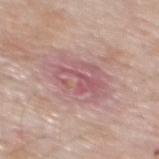Assessment:
This lesion was catalogued during total-body skin photography and was not selected for biopsy.
Clinical summary:
Cropped from a total-body skin-imaging series; the visible field is about 15 mm. A male subject, about 60 years old. Automated tile analysis of the lesion measured a footprint of about 19 mm², a shape eccentricity near 0.7, and a shape-asymmetry score of about 0.2 (0 = symmetric). It also reported a lesion–skin lightness drop of about 8 and a lesion-to-skin contrast of about 6 (normalized; higher = more distinct). The analysis additionally found a border-irregularity index near 2.5/10 and a within-lesion color-variation index near 7/10. The analysis additionally found a nevus-likeness score of about 0/100 and a lesion-detection confidence of about 95/100. From the mid back.